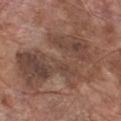| feature | finding |
|---|---|
| workup | catalogued during a skin exam; not biopsied |
| subject | male, aged 63–67 |
| location | the chest |
| tile lighting | white-light |
| image source | 15 mm crop, total-body photography |
| automated metrics | an area of roughly 50 mm², an eccentricity of roughly 0.75, and a symmetry-axis asymmetry near 0.45; a border-irregularity rating of about 8.5/10, a within-lesion color-variation index near 6.5/10, and radial color variation of about 2.5 |